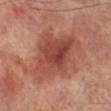Clinical impression:
The lesion was photographed on a routine skin check and not biopsied; there is no pathology result.
Background:
The total-body-photography lesion software estimated an area of roughly 31 mm² and an outline eccentricity of about 0.8 (0 = round, 1 = elongated). The analysis additionally found border irregularity of about 4 on a 0–10 scale, a color-variation rating of about 5.5/10, and a peripheral color-asymmetry measure near 2. The software also gave a classifier nevus-likeness of about 70/100 and lesion-presence confidence of about 100/100. A close-up tile cropped from a whole-body skin photograph, about 15 mm across. Longest diameter approximately 8.5 mm. A male subject aged 68–72. On the leg.